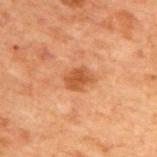acquisition=total-body-photography crop, ~15 mm field of view; body site=the upper back; patient=male, aged 68 to 72.